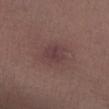Imaged during a routine full-body skin examination; the lesion was not biopsied and no histopathology is available.
Captured under white-light illumination.
The subject is a male approximately 55 years of age.
Located on the leg.
Longest diameter approximately 3 mm.
The total-body-photography lesion software estimated an area of roughly 5.5 mm², a shape eccentricity near 0.65, and a symmetry-axis asymmetry near 0.2. The analysis additionally found a border-irregularity index near 2/10 and radial color variation of about 0.5.
Cropped from a whole-body photographic skin survey; the tile spans about 15 mm.